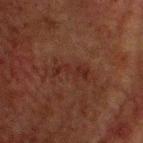Imaged during a routine full-body skin examination; the lesion was not biopsied and no histopathology is available. A 15 mm crop from a total-body photograph taken for skin-cancer surveillance. Located on the head or neck. The subject is a male aged 58–62.